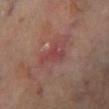Cropped from a whole-body photographic skin survey; the tile spans about 15 mm. Measured at roughly 3 mm in maximum diameter. The lesion-visualizer software estimated a classifier nevus-likeness of about 0/100. From the left lower leg. This is a cross-polarized tile. The patient is a male approximately 70 years of age.